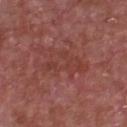Case summary:
- workup — imaged on a skin check; not biopsied
- TBP lesion metrics — a lesion area of about 12 mm² and a symmetry-axis asymmetry near 0.45; an average lesion color of about L≈40 a*≈26 b*≈25 (CIELAB) and a lesion–skin lightness drop of about 5; an automated nevus-likeness rating near 0 out of 100
- lesion size — ~6 mm (longest diameter)
- subject — male, about 65 years old
- acquisition — total-body-photography crop, ~15 mm field of view
- body site — the chest